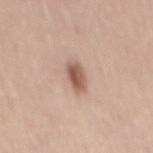biopsy status = catalogued during a skin exam; not biopsied | imaging modality = ~15 mm tile from a whole-body skin photo | anatomic site = the mid back | lesion size = about 3.5 mm | subject = male, aged approximately 45 | illumination = white-light illumination | automated metrics = an average lesion color of about L≈57 a*≈19 b*≈27 (CIELAB), about 13 CIELAB-L* units darker than the surrounding skin, and a normalized lesion–skin contrast near 8.5; border irregularity of about 3 on a 0–10 scale, a within-lesion color-variation index near 4.5/10, and a peripheral color-asymmetry measure near 1.5; a nevus-likeness score of about 95/100 and lesion-presence confidence of about 100/100.